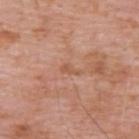Assessment: The lesion was tiled from a total-body skin photograph and was not biopsied. Acquisition and patient details: The tile uses white-light illumination. A male subject, about 60 years old. A 15 mm close-up extracted from a 3D total-body photography capture. The lesion's longest dimension is about 2.5 mm. The lesion is located on the upper back.